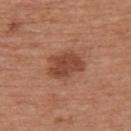<tbp_lesion>
<biopsy_status>not biopsied; imaged during a skin examination</biopsy_status>
</tbp_lesion>